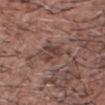The lesion was photographed on a routine skin check and not biopsied; there is no pathology result. A male patient, aged 53 to 57. A region of skin cropped from a whole-body photographic capture, roughly 15 mm wide. The recorded lesion diameter is about 3 mm. The lesion is on the head or neck.